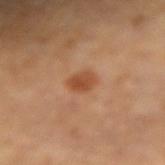The lesion was photographed on a routine skin check and not biopsied; there is no pathology result.
This is a cross-polarized tile.
Automated tile analysis of the lesion measured an average lesion color of about L≈47 a*≈24 b*≈35 (CIELAB), a lesion–skin lightness drop of about 10, and a lesion-to-skin contrast of about 8 (normalized; higher = more distinct). And it measured a nevus-likeness score of about 85/100 and lesion-presence confidence of about 100/100.
Longest diameter approximately 2.5 mm.
Cropped from a whole-body photographic skin survey; the tile spans about 15 mm.
The lesion is located on the arm.
A male subject, roughly 85 years of age or older.